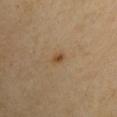Imaged during a routine full-body skin examination; the lesion was not biopsied and no histopathology is available. A male patient roughly 70 years of age. From the left upper arm. Captured under cross-polarized illumination. Cropped from a whole-body photographic skin survey; the tile spans about 15 mm. About 2 mm across.